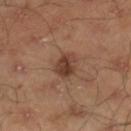Part of a total-body skin-imaging series; this lesion was reviewed on a skin check and was not flagged for biopsy. About 3.5 mm across. The tile uses cross-polarized illumination. A region of skin cropped from a whole-body photographic capture, roughly 15 mm wide. From the right lower leg. The subject is a male aged around 45. The total-body-photography lesion software estimated a within-lesion color-variation index near 4.5/10 and peripheral color asymmetry of about 1.5.